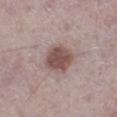Impression: Recorded during total-body skin imaging; not selected for excision or biopsy. Clinical summary: A male subject, aged approximately 75. Cropped from a total-body skin-imaging series; the visible field is about 15 mm. The lesion is located on the left lower leg.